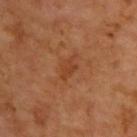{"biopsy_status": "not biopsied; imaged during a skin examination", "lesion_size": {"long_diameter_mm_approx": 3.0}, "patient": {"sex": "male", "age_approx": 65}, "image": {"source": "total-body photography crop", "field_of_view_mm": 15}, "lighting": "cross-polarized", "site": "upper back"}